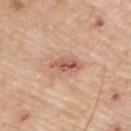Part of a total-body skin-imaging series; this lesion was reviewed on a skin check and was not flagged for biopsy.
About 4.5 mm across.
A region of skin cropped from a whole-body photographic capture, roughly 15 mm wide.
Captured under white-light illumination.
On the right upper arm.
The subject is a male aged 63 to 67.